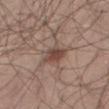{
  "biopsy_status": "not biopsied; imaged during a skin examination",
  "automated_metrics": {
    "eccentricity": 0.7,
    "shape_asymmetry": 0.25
  },
  "lighting": "white-light",
  "lesion_size": {
    "long_diameter_mm_approx": 3.5
  },
  "image": {
    "source": "total-body photography crop",
    "field_of_view_mm": 15
  },
  "patient": {
    "sex": "male",
    "age_approx": 45
  },
  "site": "leg"
}